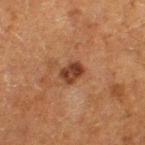Q: Is there a histopathology result?
A: imaged on a skin check; not biopsied
Q: How was the tile lit?
A: cross-polarized
Q: Where on the body is the lesion?
A: the leg
Q: Patient demographics?
A: female, aged 48 to 52
Q: What did automated image analysis measure?
A: a lesion area of about 5 mm²; a border-irregularity index near 1.5/10, a color-variation rating of about 3.5/10, and peripheral color asymmetry of about 1.5
Q: What kind of image is this?
A: 15 mm crop, total-body photography